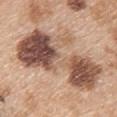Acquisition and patient details: The total-body-photography lesion software estimated a shape-asymmetry score of about 0.35 (0 = symmetric). The analysis additionally found an average lesion color of about L≈53 a*≈19 b*≈28 (CIELAB), a lesion–skin lightness drop of about 17, and a normalized border contrast of about 11. The analysis additionally found a border-irregularity rating of about 7.5/10, a color-variation rating of about 10/10, and a peripheral color-asymmetry measure near 4.5. This is a white-light tile. A region of skin cropped from a whole-body photographic capture, roughly 15 mm wide. A female subject about 45 years old. Located on the upper back. Conclusion: The lesion was biopsied, and histopathology showed a benign skin lesion: dysplastic (Clark) nevus.